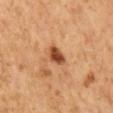workup: catalogued during a skin exam; not biopsied | image-analysis metrics: a nevus-likeness score of about 95/100 and a lesion-detection confidence of about 100/100 | anatomic site: the back | image: total-body-photography crop, ~15 mm field of view | lesion diameter: ≈2.5 mm | subject: male, approximately 60 years of age | lighting: cross-polarized illumination.